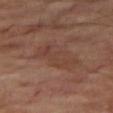Captured during whole-body skin photography for melanoma surveillance; the lesion was not biopsied. About 5.5 mm across. A male subject, aged approximately 70. Captured under cross-polarized illumination. A close-up tile cropped from a whole-body skin photograph, about 15 mm across. The lesion is on the leg.